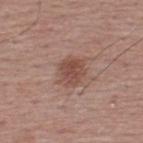A male patient aged 38 to 42. Captured under white-light illumination. A region of skin cropped from a whole-body photographic capture, roughly 15 mm wide. The recorded lesion diameter is about 3.5 mm. The lesion is on the upper back.